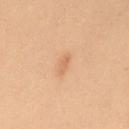Part of a total-body skin-imaging series; this lesion was reviewed on a skin check and was not flagged for biopsy. A 15 mm crop from a total-body photograph taken for skin-cancer surveillance. Imaged with cross-polarized lighting. The lesion's longest dimension is about 2.5 mm. A female patient aged 38 to 42. The lesion is on the abdomen.